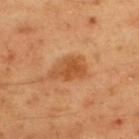Q: Was this lesion biopsied?
A: total-body-photography surveillance lesion; no biopsy
Q: What are the patient's age and sex?
A: male, aged 43 to 47
Q: How was this image acquired?
A: ~15 mm tile from a whole-body skin photo
Q: Lesion size?
A: ≈4.5 mm
Q: What lighting was used for the tile?
A: cross-polarized
Q: Automated lesion metrics?
A: a lesion–skin lightness drop of about 10 and a normalized lesion–skin contrast near 7; a detector confidence of about 100 out of 100 that the crop contains a lesion
Q: Lesion location?
A: the upper back